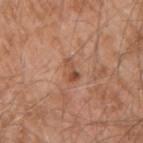Clinical impression: This lesion was catalogued during total-body skin photography and was not selected for biopsy. Acquisition and patient details: A 15 mm close-up tile from a total-body photography series done for melanoma screening. On the left upper arm. The subject is a male aged 58 to 62.